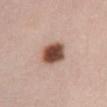Imaged during a routine full-body skin examination; the lesion was not biopsied and no histopathology is available. This is a white-light tile. Measured at roughly 4 mm in maximum diameter. A female patient roughly 50 years of age. The lesion is on the front of the torso. A lesion tile, about 15 mm wide, cut from a 3D total-body photograph.